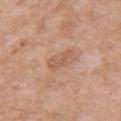Clinical impression: Captured during whole-body skin photography for melanoma surveillance; the lesion was not biopsied. Clinical summary: A roughly 15 mm field-of-view crop from a total-body skin photograph. The lesion is on the left upper arm. A female subject aged around 55. The lesion's longest dimension is about 3 mm. This is a white-light tile.